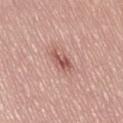Recorded during total-body skin imaging; not selected for excision or biopsy.